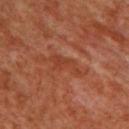Assessment:
Part of a total-body skin-imaging series; this lesion was reviewed on a skin check and was not flagged for biopsy.
Acquisition and patient details:
This is a cross-polarized tile. The subject is a male aged around 60. A close-up tile cropped from a whole-body skin photograph, about 15 mm across. The lesion is located on the chest. About 4.5 mm across. Automated tile analysis of the lesion measured a footprint of about 6.5 mm² and a shape-asymmetry score of about 0.45 (0 = symmetric). And it measured a lesion–skin lightness drop of about 5 and a normalized lesion–skin contrast near 5. It also reported an automated nevus-likeness rating near 0 out of 100 and a detector confidence of about 60 out of 100 that the crop contains a lesion.